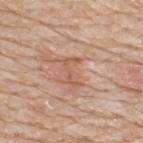The lesion's longest dimension is about 3.5 mm.
The patient is a male aged 78–82.
Imaged with white-light lighting.
From the upper back.
This image is a 15 mm lesion crop taken from a total-body photograph.
Automated image analysis of the tile measured a lesion color around L≈57 a*≈22 b*≈31 in CIELAB, about 8 CIELAB-L* units darker than the surrounding skin, and a normalized border contrast of about 5.5. The analysis additionally found border irregularity of about 9 on a 0–10 scale. It also reported a nevus-likeness score of about 0/100 and a detector confidence of about 95 out of 100 that the crop contains a lesion.